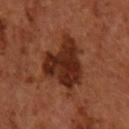* follow-up · catalogued during a skin exam; not biopsied
* lesion size · ~6.5 mm (longest diameter)
* anatomic site · the right forearm
* tile lighting · cross-polarized
* patient · female, aged 63–67
* image · ~15 mm tile from a whole-body skin photo